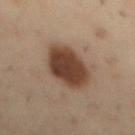| field | value |
|---|---|
| follow-up | total-body-photography surveillance lesion; no biopsy |
| tile lighting | cross-polarized illumination |
| acquisition | ~15 mm tile from a whole-body skin photo |
| site | the mid back |
| subject | male, aged around 55 |